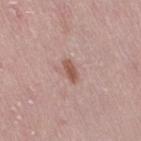* notes: total-body-photography surveillance lesion; no biopsy
* image source: ~15 mm crop, total-body skin-cancer survey
* subject: female, approximately 50 years of age
* image-analysis metrics: a footprint of about 2.5 mm² and a symmetry-axis asymmetry near 0.3; a lesion color around L≈53 a*≈22 b*≈26 in CIELAB and a normalized border contrast of about 8.5; border irregularity of about 3 on a 0–10 scale and a peripheral color-asymmetry measure near 0
* illumination: white-light
* site: the right thigh